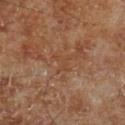Q: Was this lesion biopsied?
A: total-body-photography surveillance lesion; no biopsy
Q: What did automated image analysis measure?
A: a shape-asymmetry score of about 0.6 (0 = symmetric); a mean CIELAB color near L≈43 a*≈20 b*≈32, about 4 CIELAB-L* units darker than the surrounding skin, and a normalized border contrast of about 4.5; a color-variation rating of about 0/10 and a peripheral color-asymmetry measure near 0; a classifier nevus-likeness of about 0/100 and a detector confidence of about 55 out of 100 that the crop contains a lesion
Q: What is the lesion's diameter?
A: ≈2.5 mm
Q: How was this image acquired?
A: ~15 mm crop, total-body skin-cancer survey
Q: Where on the body is the lesion?
A: the left lower leg
Q: Patient demographics?
A: male, aged approximately 70
Q: What lighting was used for the tile?
A: cross-polarized illumination